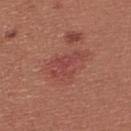Recorded during total-body skin imaging; not selected for excision or biopsy.
A 15 mm close-up tile from a total-body photography series done for melanoma screening.
Imaged with white-light lighting.
Located on the upper back.
The lesion's longest dimension is about 4.5 mm.
A female subject aged 23–27.